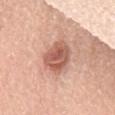Case summary:
– follow-up · no biopsy performed (imaged during a skin exam)
– tile lighting · white-light
– anatomic site · the upper back
– diameter · about 5 mm
– patient · male, about 65 years old
– automated metrics · a mean CIELAB color near L≈58 a*≈24 b*≈29, a lesion–skin lightness drop of about 13, and a normalized border contrast of about 8.5; a border-irregularity rating of about 2.5/10, a color-variation rating of about 5/10, and a peripheral color-asymmetry measure near 1.5
– image · ~15 mm crop, total-body skin-cancer survey